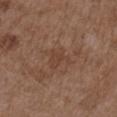Case summary:
- workup: catalogued during a skin exam; not biopsied
- patient: female, in their mid-30s
- image: total-body-photography crop, ~15 mm field of view
- tile lighting: white-light
- site: the right upper arm
- size: ~2.5 mm (longest diameter)
- automated metrics: a mean CIELAB color near L≈41 a*≈19 b*≈27, a lesion–skin lightness drop of about 5, and a normalized border contrast of about 4.5; a border-irregularity rating of about 4.5/10, internal color variation of about 1.5 on a 0–10 scale, and a peripheral color-asymmetry measure near 0.5